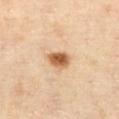notes: catalogued during a skin exam; not biopsied
patient: female, aged 43 to 47
automated metrics: a border-irregularity index near 2/10 and internal color variation of about 5 on a 0–10 scale; a classifier nevus-likeness of about 100/100 and a detector confidence of about 100 out of 100 that the crop contains a lesion
location: the abdomen
image source: 15 mm crop, total-body photography
illumination: cross-polarized illumination